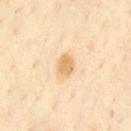This lesion was catalogued during total-body skin photography and was not selected for biopsy. Captured under cross-polarized illumination. On the chest. The patient is a male roughly 45 years of age. The recorded lesion diameter is about 2.5 mm. A region of skin cropped from a whole-body photographic capture, roughly 15 mm wide.